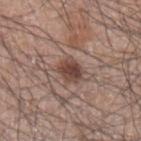follow-up = total-body-photography surveillance lesion; no biopsy
lesion size = about 3 mm
patient = male, in their 70s
lighting = white-light
imaging modality = ~15 mm tile from a whole-body skin photo
site = the left upper arm